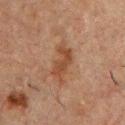Findings:
* biopsy status: catalogued during a skin exam; not biopsied
* image source: total-body-photography crop, ~15 mm field of view
* image-analysis metrics: border irregularity of about 5 on a 0–10 scale, a color-variation rating of about 2/10, and peripheral color asymmetry of about 0.5
* lesion diameter: about 5 mm
* site: the chest
* subject: male, approximately 50 years of age